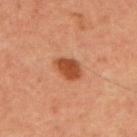Captured during whole-body skin photography for melanoma surveillance; the lesion was not biopsied.